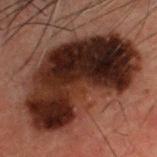workup = total-body-photography surveillance lesion; no biopsy | subject = male, approximately 50 years of age | imaging modality = ~15 mm crop, total-body skin-cancer survey | automated metrics = a border-irregularity rating of about 3.5/10, internal color variation of about 10 on a 0–10 scale, and radial color variation of about 3.5; a classifier nevus-likeness of about 5/100 and lesion-presence confidence of about 100/100 | location = the head or neck | lighting = cross-polarized illumination | size = ~12.5 mm (longest diameter).